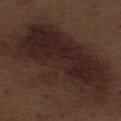The lesion was photographed on a routine skin check and not biopsied; there is no pathology result. About 16.5 mm across. From the left thigh. The total-body-photography lesion software estimated a footprint of about 80 mm², an outline eccentricity of about 0.9 (0 = round, 1 = elongated), and two-axis asymmetry of about 0.3. The patient is a male aged approximately 70. Imaged with white-light lighting. A roughly 15 mm field-of-view crop from a total-body skin photograph.